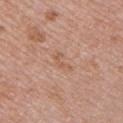| key | value |
|---|---|
| acquisition | 15 mm crop, total-body photography |
| anatomic site | the chest |
| patient | female, approximately 50 years of age |